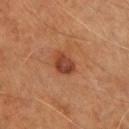The lesion was photographed on a routine skin check and not biopsied; there is no pathology result.
A region of skin cropped from a whole-body photographic capture, roughly 15 mm wide.
The lesion is on the chest.
Longest diameter approximately 3 mm.
Imaged with cross-polarized lighting.
The subject is a male in their mid-50s.